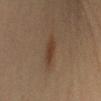{
  "biopsy_status": "not biopsied; imaged during a skin examination",
  "site": "left upper arm",
  "image": {
    "source": "total-body photography crop",
    "field_of_view_mm": 15
  },
  "lighting": "cross-polarized",
  "patient": {
    "sex": "female",
    "age_approx": 45
  },
  "lesion_size": {
    "long_diameter_mm_approx": 4.0
  },
  "automated_metrics": {
    "area_mm2_approx": 6.0,
    "eccentricity": 0.85,
    "shape_asymmetry": 0.2,
    "border_irregularity_0_10": 2.5,
    "color_variation_0_10": 2.0,
    "peripheral_color_asymmetry": 0.5,
    "nevus_likeness_0_100": 90,
    "lesion_detection_confidence_0_100": 100
  }
}